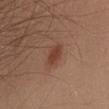{"biopsy_status": "not biopsied; imaged during a skin examination", "lighting": "white-light", "lesion_size": {"long_diameter_mm_approx": 3.0}, "automated_metrics": {"area_mm2_approx": 5.0, "eccentricity": 0.75, "shape_asymmetry": 0.2, "border_irregularity_0_10": 2.0, "color_variation_0_10": 2.0, "peripheral_color_asymmetry": 0.5}, "image": {"source": "total-body photography crop", "field_of_view_mm": 15}, "patient": {"sex": "male", "age_approx": 35}, "site": "chest"}